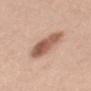Captured during whole-body skin photography for melanoma surveillance; the lesion was not biopsied. A lesion tile, about 15 mm wide, cut from a 3D total-body photograph. The lesion's longest dimension is about 5.5 mm. A male patient aged approximately 50. The lesion is located on the mid back.